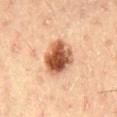Assessment:
Captured during whole-body skin photography for melanoma surveillance; the lesion was not biopsied.
Context:
Cropped from a whole-body photographic skin survey; the tile spans about 15 mm. A male patient approximately 50 years of age. From the mid back. An algorithmic analysis of the crop reported border irregularity of about 1.5 on a 0–10 scale and internal color variation of about 7.5 on a 0–10 scale. The tile uses cross-polarized illumination.